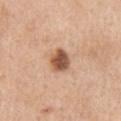Findings:
- notes — catalogued during a skin exam; not biopsied
- site — the right upper arm
- imaging modality — total-body-photography crop, ~15 mm field of view
- subject — female, aged 53 to 57
- automated lesion analysis — an automated nevus-likeness rating near 95 out of 100
- lighting — white-light